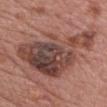Findings:
- biopsy status · catalogued during a skin exam; not biopsied
- site · the chest
- subject · female, approximately 60 years of age
- lesion diameter · about 10.5 mm
- image · ~15 mm tile from a whole-body skin photo